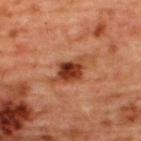| feature | finding |
|---|---|
| notes | imaged on a skin check; not biopsied |
| site | the back |
| imaging modality | ~15 mm crop, total-body skin-cancer survey |
| patient | female, aged around 45 |
| TBP lesion metrics | an average lesion color of about L≈42 a*≈28 b*≈34 (CIELAB), a lesion–skin lightness drop of about 13, and a normalized border contrast of about 10; a nevus-likeness score of about 90/100 and a lesion-detection confidence of about 100/100 |
| illumination | cross-polarized illumination |